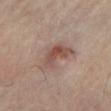Findings:
• subject — female, aged approximately 65
• TBP lesion metrics — a footprint of about 10 mm² and a symmetry-axis asymmetry near 0.45; a mean CIELAB color near L≈52 a*≈19 b*≈24 and a lesion-to-skin contrast of about 7 (normalized; higher = more distinct); a within-lesion color-variation index near 5/10 and peripheral color asymmetry of about 1.5; a lesion-detection confidence of about 100/100
• image — total-body-photography crop, ~15 mm field of view
• lighting — cross-polarized
• anatomic site — the left lower leg
• lesion diameter — about 5.5 mm